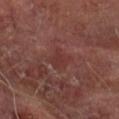The lesion was tiled from a total-body skin photograph and was not biopsied.
About 2.5 mm across.
A close-up tile cropped from a whole-body skin photograph, about 15 mm across.
The subject is a male aged around 65.
The lesion is on the right forearm.
Captured under cross-polarized illumination.